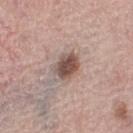Clinical impression: This lesion was catalogued during total-body skin photography and was not selected for biopsy. Clinical summary: The recorded lesion diameter is about 3 mm. The lesion is on the left thigh. A roughly 15 mm field-of-view crop from a total-body skin photograph. The patient is a female aged 63–67.